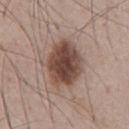| feature | finding |
|---|---|
| follow-up | total-body-photography surveillance lesion; no biopsy |